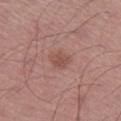Impression:
This lesion was catalogued during total-body skin photography and was not selected for biopsy.
Background:
A 15 mm crop from a total-body photograph taken for skin-cancer surveillance. The lesion is located on the right lower leg. The patient is a male aged 63 to 67.